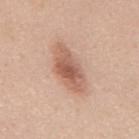Context: Imaged with white-light lighting. About 6 mm across. A 15 mm close-up tile from a total-body photography series done for melanoma screening. A male patient, approximately 50 years of age. On the mid back.